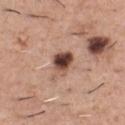{
  "biopsy_status": "not biopsied; imaged during a skin examination",
  "patient": {
    "sex": "male",
    "age_approx": 45
  },
  "site": "front of the torso",
  "image": {
    "source": "total-body photography crop",
    "field_of_view_mm": 15
  },
  "lesion_size": {
    "long_diameter_mm_approx": 3.0
  },
  "lighting": "white-light"
}